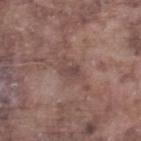location: the right lower leg
automated lesion analysis: an area of roughly 4.5 mm², an outline eccentricity of about 0.75 (0 = round, 1 = elongated), and a symmetry-axis asymmetry near 0.2; a classifier nevus-likeness of about 0/100 and a lesion-detection confidence of about 95/100
image: total-body-photography crop, ~15 mm field of view
tile lighting: white-light
lesion diameter: ≈3 mm
subject: male, approximately 75 years of age